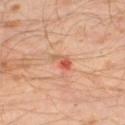Clinical impression: Part of a total-body skin-imaging series; this lesion was reviewed on a skin check and was not flagged for biopsy. Acquisition and patient details: The tile uses cross-polarized illumination. The lesion is on the left thigh. A lesion tile, about 15 mm wide, cut from a 3D total-body photograph. A male subject about 30 years old.